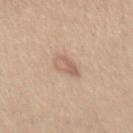The lesion was tiled from a total-body skin photograph and was not biopsied.
The lesion is located on the abdomen.
Cropped from a total-body skin-imaging series; the visible field is about 15 mm.
Automated image analysis of the tile measured a classifier nevus-likeness of about 5/100 and a lesion-detection confidence of about 100/100.
This is a white-light tile.
A female patient in their mid-40s.
Approximately 3.5 mm at its widest.